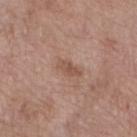  automated_metrics:
    area_mm2_approx: 4.0
    eccentricity: 0.85
    shape_asymmetry: 0.3
    cielab_L: 52
    cielab_a: 20
    cielab_b: 28
    vs_skin_darker_L: 8.0
    vs_skin_contrast_norm: 6.0
    nevus_likeness_0_100: 0
    lesion_detection_confidence_0_100: 100
  lighting: white-light
  site: leg
  lesion_size:
    long_diameter_mm_approx: 3.0
  image:
    source: total-body photography crop
    field_of_view_mm: 15
  patient:
    sex: female
    age_approx: 70